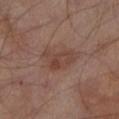| key | value |
|---|---|
| workup | imaged on a skin check; not biopsied |
| patient | male, aged 63 to 67 |
| image source | 15 mm crop, total-body photography |
| lighting | cross-polarized illumination |
| location | the left lower leg |